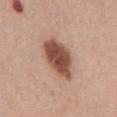Clinical impression:
Captured during whole-body skin photography for melanoma surveillance; the lesion was not biopsied.
Acquisition and patient details:
An algorithmic analysis of the crop reported a lesion color around L≈50 a*≈21 b*≈28 in CIELAB, a lesion–skin lightness drop of about 17, and a lesion-to-skin contrast of about 11.5 (normalized; higher = more distinct). Approximately 6 mm at its widest. A 15 mm crop from a total-body photograph taken for skin-cancer surveillance. A female subject, aged approximately 60. The lesion is located on the abdomen.